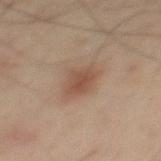Findings:
* workup · total-body-photography surveillance lesion; no biopsy
* body site · the back
* lighting · cross-polarized
* subject · male, in their 60s
* diameter · ≈4 mm
* image source · ~15 mm crop, total-body skin-cancer survey